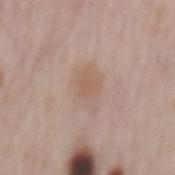Clinical impression:
Imaged during a routine full-body skin examination; the lesion was not biopsied and no histopathology is available.
Clinical summary:
Cropped from a total-body skin-imaging series; the visible field is about 15 mm. On the mid back. A male patient, about 65 years old.